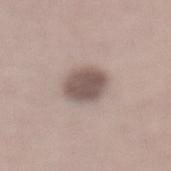Findings:
• biopsy status · imaged on a skin check; not biopsied
• acquisition · ~15 mm crop, total-body skin-cancer survey
• patient · male, in their 70s
• anatomic site · the lower back
• lesion diameter · ≈3.5 mm
• tile lighting · white-light